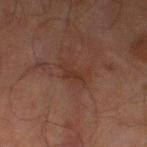This lesion was catalogued during total-body skin photography and was not selected for biopsy. Cropped from a total-body skin-imaging series; the visible field is about 15 mm. A male subject about 70 years old. Located on the left thigh. This is a cross-polarized tile. Longest diameter approximately 3 mm.